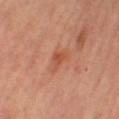Q: What is the anatomic site?
A: the right thigh
Q: What kind of image is this?
A: total-body-photography crop, ~15 mm field of view
Q: What did automated image analysis measure?
A: an area of roughly 4 mm², an eccentricity of roughly 0.75, and a shape-asymmetry score of about 0.45 (0 = symmetric); a border-irregularity index near 4/10, a within-lesion color-variation index near 3/10, and a peripheral color-asymmetry measure near 1; an automated nevus-likeness rating near 0 out of 100 and a lesion-detection confidence of about 100/100
Q: What are the patient's age and sex?
A: female, in their mid- to late 60s
Q: Illumination type?
A: cross-polarized
Q: Lesion size?
A: about 3 mm